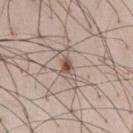The lesion was tiled from a total-body skin photograph and was not biopsied. From the right thigh. This is a white-light tile. A 15 mm crop from a total-body photograph taken for skin-cancer surveillance. The patient is a male aged approximately 35. Measured at roughly 4 mm in maximum diameter. Automated tile analysis of the lesion measured about 11 CIELAB-L* units darker than the surrounding skin. The analysis additionally found an automated nevus-likeness rating near 45 out of 100 and a lesion-detection confidence of about 90/100.